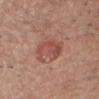| key | value |
|---|---|
| notes | no biopsy performed (imaged during a skin exam) |
| acquisition | 15 mm crop, total-body photography |
| body site | the head or neck |
| patient | male, aged 43–47 |
| tile lighting | white-light illumination |